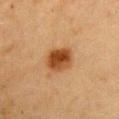Captured during whole-body skin photography for melanoma surveillance; the lesion was not biopsied. Located on the chest. Cropped from a whole-body photographic skin survey; the tile spans about 15 mm. A female subject, in their mid-50s.